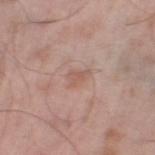Case summary:
* workup: no biopsy performed (imaged during a skin exam)
* location: the left lower leg
* TBP lesion metrics: a lesion area of about 3.5 mm², an outline eccentricity of about 0.75 (0 = round, 1 = elongated), and a symmetry-axis asymmetry near 0.4
* image: ~15 mm crop, total-body skin-cancer survey
* subject: male, aged 53 to 57
* lesion size: ~2.5 mm (longest diameter)